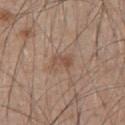<record>
<biopsy_status>not biopsied; imaged during a skin examination</biopsy_status>
<automated_metrics>
  <area_mm2_approx>6.5</area_mm2_approx>
  <eccentricity>0.6</eccentricity>
  <shape_asymmetry>0.2</shape_asymmetry>
  <cielab_L>52</cielab_L>
  <cielab_a>17</cielab_a>
  <cielab_b>27</cielab_b>
  <vs_skin_darker_L>7.0</vs_skin_darker_L>
  <vs_skin_contrast_norm>5.5</vs_skin_contrast_norm>
  <lesion_detection_confidence_0_100>100</lesion_detection_confidence_0_100>
</automated_metrics>
<lighting>white-light</lighting>
<image>
  <source>total-body photography crop</source>
  <field_of_view_mm>15</field_of_view_mm>
</image>
<patient>
  <sex>male</sex>
  <age_approx>50</age_approx>
</patient>
<site>upper back</site>
<lesion_size>
  <long_diameter_mm_approx>3.0</long_diameter_mm_approx>
</lesion_size>
</record>